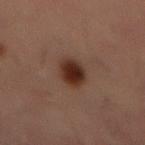A male subject, roughly 55 years of age.
The lesion-visualizer software estimated an area of roughly 7 mm², an eccentricity of roughly 0.7, and a shape-asymmetry score of about 0.1 (0 = symmetric). The analysis additionally found a mean CIELAB color near L≈25 a*≈17 b*≈22 and a lesion-to-skin contrast of about 12 (normalized; higher = more distinct). And it measured radial color variation of about 1.
A roughly 15 mm field-of-view crop from a total-body skin photograph.
Located on the abdomen.
About 3.5 mm across.